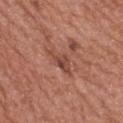follow-up: total-body-photography surveillance lesion; no biopsy
imaging modality: ~15 mm crop, total-body skin-cancer survey
patient: female, in their 60s
site: the upper back